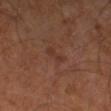The lesion was photographed on a routine skin check and not biopsied; there is no pathology result.
The lesion is located on the left thigh.
Captured under cross-polarized illumination.
Approximately 3 mm at its widest.
Automated image analysis of the tile measured a classifier nevus-likeness of about 0/100 and a lesion-detection confidence of about 100/100.
A 15 mm close-up extracted from a 3D total-body photography capture.
A male patient in their mid- to late 60s.